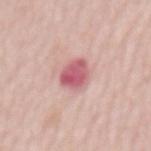Recorded during total-body skin imaging; not selected for excision or biopsy.
A female subject, roughly 65 years of age.
Automated tile analysis of the lesion measured a footprint of about 8 mm², an outline eccentricity of about 0.75 (0 = round, 1 = elongated), and two-axis asymmetry of about 0.15. And it measured an average lesion color of about L≈59 a*≈31 b*≈19 (CIELAB), a lesion–skin lightness drop of about 14, and a normalized border contrast of about 9. And it measured border irregularity of about 2 on a 0–10 scale and internal color variation of about 4.5 on a 0–10 scale. The analysis additionally found an automated nevus-likeness rating near 0 out of 100.
The lesion's longest dimension is about 4 mm.
Cropped from a whole-body photographic skin survey; the tile spans about 15 mm.
From the mid back.